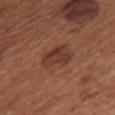workup = imaged on a skin check; not biopsied | acquisition = ~15 mm crop, total-body skin-cancer survey | site = the chest | tile lighting = white-light | subject = female, in their mid-60s.